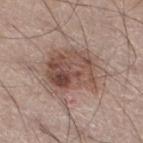No biopsy was performed on this lesion — it was imaged during a full skin examination and was not determined to be concerning. The total-body-photography lesion software estimated a border-irregularity rating of about 4/10 and internal color variation of about 8 on a 0–10 scale. A male patient, about 50 years old. A roughly 15 mm field-of-view crop from a total-body skin photograph. Approximately 7 mm at its widest. The lesion is located on the left lower leg. Captured under white-light illumination.